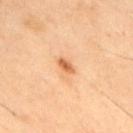<case>
<biopsy_status>not biopsied; imaged during a skin examination</biopsy_status>
<patient>
  <sex>male</sex>
  <age_approx>45</age_approx>
</patient>
<site>back</site>
<image>
  <source>total-body photography crop</source>
  <field_of_view_mm>15</field_of_view_mm>
</image>
</case>